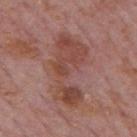Impression: No biopsy was performed on this lesion — it was imaged during a full skin examination and was not determined to be concerning. Background: The tile uses white-light illumination. Located on the mid back. A lesion tile, about 15 mm wide, cut from a 3D total-body photograph. A male subject approximately 75 years of age. The lesion's longest dimension is about 8 mm. An algorithmic analysis of the crop reported a lesion color around L≈45 a*≈23 b*≈26 in CIELAB, about 9 CIELAB-L* units darker than the surrounding skin, and a lesion-to-skin contrast of about 7 (normalized; higher = more distinct). The software also gave a border-irregularity rating of about 8.5/10, internal color variation of about 4 on a 0–10 scale, and radial color variation of about 1.